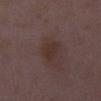follow-up = total-body-photography surveillance lesion; no biopsy
body site = the right lower leg
lesion diameter = about 2.5 mm
imaging modality = ~15 mm crop, total-body skin-cancer survey
subject = female, aged 28–32